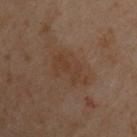workup = imaged on a skin check; not biopsied | illumination = cross-polarized illumination | site = the upper back | subject = male, aged approximately 50 | imaging modality = ~15 mm tile from a whole-body skin photo | automated lesion analysis = an area of roughly 11 mm² and two-axis asymmetry of about 0.25; a lesion color around L≈30 a*≈13 b*≈23 in CIELAB, roughly 4 lightness units darker than nearby skin, and a normalized lesion–skin contrast near 5; border irregularity of about 3.5 on a 0–10 scale and a peripheral color-asymmetry measure near 1 | size = about 5 mm.